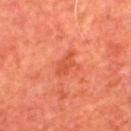The lesion was photographed on a routine skin check and not biopsied; there is no pathology result. The lesion-visualizer software estimated a mean CIELAB color near L≈49 a*≈36 b*≈38 and a normalized border contrast of about 5.5. It also reported a nevus-likeness score of about 5/100 and a lesion-detection confidence of about 100/100. On the back. A 15 mm close-up extracted from a 3D total-body photography capture. A male patient, in their mid-60s.